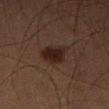{"biopsy_status": "not biopsied; imaged during a skin examination", "patient": {"sex": "male", "age_approx": 65}, "lighting": "cross-polarized", "site": "leg", "lesion_size": {"long_diameter_mm_approx": 3.0}, "image": {"source": "total-body photography crop", "field_of_view_mm": 15}, "automated_metrics": {"area_mm2_approx": 6.5, "eccentricity": 0.35, "shape_asymmetry": 0.2, "cielab_L": 16, "cielab_a": 13, "cielab_b": 16, "vs_skin_darker_L": 8.0, "vs_skin_contrast_norm": 10.5, "lesion_detection_confidence_0_100": 100}}